The lesion is located on the mid back, cropped from a whole-body photographic skin survey; the tile spans about 15 mm, a male subject in their mid-60s.
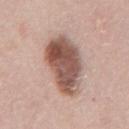Histopathology of the biopsied lesion showed a dysplastic (Clark) nevus — a benign lesion.